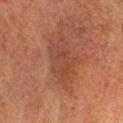The patient is a male roughly 65 years of age. From the head or neck. Captured under cross-polarized illumination. Automated image analysis of the tile measured a mean CIELAB color near L≈35 a*≈21 b*≈26, about 5 CIELAB-L* units darker than the surrounding skin, and a normalized lesion–skin contrast near 5. And it measured border irregularity of about 4 on a 0–10 scale and a color-variation rating of about 3/10. A region of skin cropped from a whole-body photographic capture, roughly 15 mm wide.